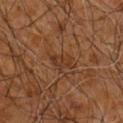<record>
<biopsy_status>not biopsied; imaged during a skin examination</biopsy_status>
<lighting>cross-polarized</lighting>
<site>arm</site>
<lesion_size>
  <long_diameter_mm_approx>2.5</long_diameter_mm_approx>
</lesion_size>
<image>
  <source>total-body photography crop</source>
  <field_of_view_mm>15</field_of_view_mm>
</image>
<automated_metrics>
  <border_irregularity_0_10>3.0</border_irregularity_0_10>
  <color_variation_0_10>1.5</color_variation_0_10>
  <peripheral_color_asymmetry>0.5</peripheral_color_asymmetry>
</automated_metrics>
<patient>
  <sex>male</sex>
  <age_approx>60</age_approx>
</patient>
</record>